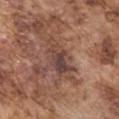Recorded during total-body skin imaging; not selected for excision or biopsy.
A male patient approximately 75 years of age.
From the left upper arm.
This image is a 15 mm lesion crop taken from a total-body photograph.